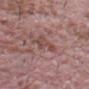<case>
  <biopsy_status>not biopsied; imaged during a skin examination</biopsy_status>
  <site>front of the torso</site>
  <image>
    <source>total-body photography crop</source>
    <field_of_view_mm>15</field_of_view_mm>
  </image>
  <automated_metrics>
    <border_irregularity_0_10>5.0</border_irregularity_0_10>
    <color_variation_0_10>2.5</color_variation_0_10>
    <peripheral_color_asymmetry>0.5</peripheral_color_asymmetry>
    <nevus_likeness_0_100>0</nevus_likeness_0_100>
  </automated_metrics>
  <lighting>white-light</lighting>
  <patient>
    <sex>male</sex>
    <age_approx>65</age_approx>
  </patient>
</case>